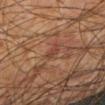Notes:
* follow-up · no biopsy performed (imaged during a skin exam)
* patient · male, aged approximately 75
* diameter · ≈2.5 mm
* lighting · cross-polarized
* anatomic site · the arm
* imaging modality · 15 mm crop, total-body photography